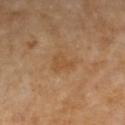Assessment:
Part of a total-body skin-imaging series; this lesion was reviewed on a skin check and was not flagged for biopsy.
Acquisition and patient details:
A 15 mm crop from a total-body photograph taken for skin-cancer surveillance. The lesion is located on the right forearm. Captured under cross-polarized illumination. The subject is a female roughly 70 years of age.